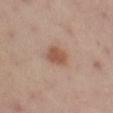The lesion was tiled from a total-body skin photograph and was not biopsied. The lesion is located on the leg. A lesion tile, about 15 mm wide, cut from a 3D total-body photograph. The patient is a female aged approximately 55. The lesion's longest dimension is about 3 mm. This is a cross-polarized tile. Automated image analysis of the tile measured an automated nevus-likeness rating near 95 out of 100 and a lesion-detection confidence of about 100/100.